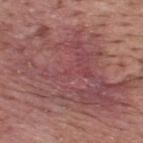A male subject in their 40s. Approximately 17.5 mm at its widest. Cropped from a total-body skin-imaging series; the visible field is about 15 mm. The lesion-visualizer software estimated a mean CIELAB color near L≈48 a*≈24 b*≈21, a lesion–skin lightness drop of about 8, and a lesion-to-skin contrast of about 6.5 (normalized; higher = more distinct). The analysis additionally found border irregularity of about 8 on a 0–10 scale, a within-lesion color-variation index near 6/10, and peripheral color asymmetry of about 2. It also reported a nevus-likeness score of about 0/100 and lesion-presence confidence of about 100/100. The lesion is on the back. The tile uses white-light illumination.